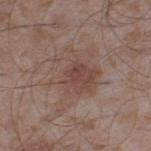notes: catalogued during a skin exam; not biopsied | location: the leg | patient: male, in their mid-40s | lighting: white-light | size: about 7 mm | imaging modality: total-body-photography crop, ~15 mm field of view.